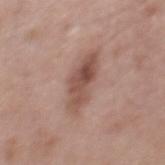Recorded during total-body skin imaging; not selected for excision or biopsy.
A region of skin cropped from a whole-body photographic capture, roughly 15 mm wide.
A male patient, aged 53 to 57.
This is a white-light tile.
Automated tile analysis of the lesion measured an average lesion color of about L≈51 a*≈20 b*≈25 (CIELAB), a lesion–skin lightness drop of about 11, and a normalized lesion–skin contrast near 8. And it measured a within-lesion color-variation index near 6/10 and radial color variation of about 2.5.
From the back.
Approximately 6 mm at its widest.